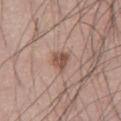Clinical impression:
Part of a total-body skin-imaging series; this lesion was reviewed on a skin check and was not flagged for biopsy.
Acquisition and patient details:
A male subject, roughly 55 years of age. Imaged with white-light lighting. Automated image analysis of the tile measured internal color variation of about 3.5 on a 0–10 scale and a peripheral color-asymmetry measure near 1.5. It also reported a nevus-likeness score of about 85/100 and a detector confidence of about 100 out of 100 that the crop contains a lesion. A 15 mm close-up extracted from a 3D total-body photography capture. Longest diameter approximately 3 mm.